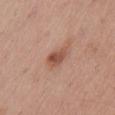biopsy status: catalogued during a skin exam; not biopsied
body site: the abdomen
patient: male, in their mid- to late 50s
illumination: white-light illumination
automated metrics: a footprint of about 5 mm², a shape eccentricity near 0.75, and a symmetry-axis asymmetry near 0.2; a lesion color around L≈52 a*≈23 b*≈29 in CIELAB, a lesion–skin lightness drop of about 11, and a lesion-to-skin contrast of about 7.5 (normalized; higher = more distinct); a border-irregularity index near 2/10 and internal color variation of about 4 on a 0–10 scale
image: ~15 mm tile from a whole-body skin photo